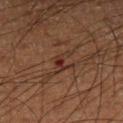Notes:
- workup — catalogued during a skin exam; not biopsied
- patient — male, aged approximately 60
- diameter — ~3 mm (longest diameter)
- lighting — cross-polarized
- imaging modality — ~15 mm crop, total-body skin-cancer survey